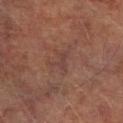Q: Is there a histopathology result?
A: catalogued during a skin exam; not biopsied
Q: What is the anatomic site?
A: the right lower leg
Q: What are the patient's age and sex?
A: male, in their mid- to late 70s
Q: What kind of image is this?
A: total-body-photography crop, ~15 mm field of view